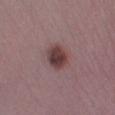biopsy status: total-body-photography surveillance lesion; no biopsy | subject: male, aged 48–52 | site: the leg | TBP lesion metrics: a shape eccentricity near 0.65 and two-axis asymmetry of about 0.2; a mean CIELAB color near L≈39 a*≈20 b*≈17 and about 13 CIELAB-L* units darker than the surrounding skin; a border-irregularity index near 2/10, a within-lesion color-variation index near 4.5/10, and peripheral color asymmetry of about 1.5; a nevus-likeness score of about 90/100 and a lesion-detection confidence of about 100/100 | acquisition: 15 mm crop, total-body photography | illumination: white-light illumination.